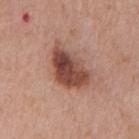Findings:
– workup — no biopsy performed (imaged during a skin exam)
– patient — male, about 70 years old
– anatomic site — the mid back
– TBP lesion metrics — a lesion area of about 15 mm² and an outline eccentricity of about 0.8 (0 = round, 1 = elongated); a border-irregularity index near 3/10, a within-lesion color-variation index near 6.5/10, and a peripheral color-asymmetry measure near 2
– diameter — ~6 mm (longest diameter)
– imaging modality — ~15 mm crop, total-body skin-cancer survey
– lighting — white-light illumination